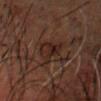Q: Is there a histopathology result?
A: total-body-photography surveillance lesion; no biopsy
Q: Lesion size?
A: ≈5.5 mm
Q: What is the anatomic site?
A: the head or neck
Q: What did automated image analysis measure?
A: a lesion area of about 9 mm², an eccentricity of roughly 0.9, and two-axis asymmetry of about 0.4; an average lesion color of about L≈17 a*≈13 b*≈17 (CIELAB), about 5 CIELAB-L* units darker than the surrounding skin, and a normalized lesion–skin contrast near 7; border irregularity of about 5 on a 0–10 scale and a color-variation rating of about 5/10
Q: Patient demographics?
A: male, aged 48–52
Q: Illumination type?
A: cross-polarized
Q: What is the imaging modality?
A: 15 mm crop, total-body photography